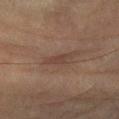Findings:
– follow-up · no biopsy performed (imaged during a skin exam)
– anatomic site · the left forearm
– subject · male, roughly 75 years of age
– image · ~15 mm tile from a whole-body skin photo